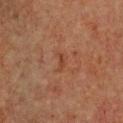notes: no biopsy performed (imaged during a skin exam); automated metrics: a nevus-likeness score of about 0/100; lighting: cross-polarized illumination; body site: the chest; image: ~15 mm crop, total-body skin-cancer survey; patient: female, about 50 years old.